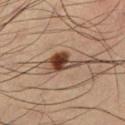Impression: The lesion was photographed on a routine skin check and not biopsied; there is no pathology result. Clinical summary: A roughly 15 mm field-of-view crop from a total-body skin photograph. The lesion is located on the left lower leg. The recorded lesion diameter is about 5 mm. A male patient in their mid- to late 30s.